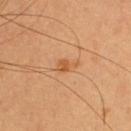follow-up: catalogued during a skin exam; not biopsied
subject: female, in their 30s
acquisition: total-body-photography crop, ~15 mm field of view
diameter: ~3 mm (longest diameter)
site: the upper back